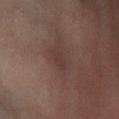notes: total-body-photography surveillance lesion; no biopsy
diameter: ~3.5 mm (longest diameter)
imaging modality: ~15 mm tile from a whole-body skin photo
location: the left lower leg
automated metrics: an average lesion color of about L≈30 a*≈15 b*≈17 (CIELAB) and a lesion-to-skin contrast of about 5.5 (normalized; higher = more distinct); a border-irregularity rating of about 3.5/10 and internal color variation of about 1 on a 0–10 scale; lesion-presence confidence of about 75/100
patient: female, aged approximately 45